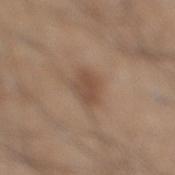<case>
<biopsy_status>not biopsied; imaged during a skin examination</biopsy_status>
<site>right lower leg</site>
<patient>
  <sex>male</sex>
  <age_approx>45</age_approx>
</patient>
<image>
  <source>total-body photography crop</source>
  <field_of_view_mm>15</field_of_view_mm>
</image>
</case>